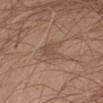| field | value |
|---|---|
| workup | total-body-photography surveillance lesion; no biopsy |
| imaging modality | ~15 mm tile from a whole-body skin photo |
| image-analysis metrics | a footprint of about 6 mm², a shape eccentricity near 0.75, and a shape-asymmetry score of about 0.3 (0 = symmetric); a mean CIELAB color near L≈49 a*≈16 b*≈27 and a lesion–skin lightness drop of about 7; a detector confidence of about 100 out of 100 that the crop contains a lesion |
| anatomic site | the front of the torso |
| subject | male, in their mid-60s |